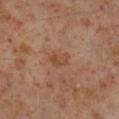No biopsy was performed on this lesion — it was imaged during a full skin examination and was not determined to be concerning.
A male patient, aged around 60.
A lesion tile, about 15 mm wide, cut from a 3D total-body photograph.
Located on the right lower leg.